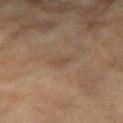Clinical impression: No biopsy was performed on this lesion — it was imaged during a full skin examination and was not determined to be concerning. Image and clinical context: The total-body-photography lesion software estimated a border-irregularity rating of about 3.5/10, internal color variation of about 0 on a 0–10 scale, and radial color variation of about 0. The lesion's longest dimension is about 2.5 mm. Located on the arm. A female subject, aged approximately 80. Captured under cross-polarized illumination. A lesion tile, about 15 mm wide, cut from a 3D total-body photograph.